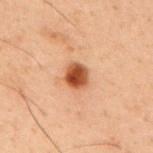Impression: Imaged during a routine full-body skin examination; the lesion was not biopsied and no histopathology is available.